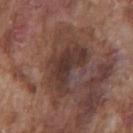Impression:
Imaged during a routine full-body skin examination; the lesion was not biopsied and no histopathology is available.
Context:
Longest diameter approximately 6.5 mm. The patient is a male aged around 75. On the front of the torso. Imaged with white-light lighting. Cropped from a whole-body photographic skin survey; the tile spans about 15 mm.